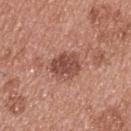Notes:
• workup · imaged on a skin check; not biopsied
• illumination · white-light
• image-analysis metrics · an area of roughly 11 mm², a shape eccentricity near 0.6, and a symmetry-axis asymmetry near 0.15; an average lesion color of about L≈49 a*≈24 b*≈27 (CIELAB), about 11 CIELAB-L* units darker than the surrounding skin, and a normalized border contrast of about 8
• location · the upper back
• acquisition · ~15 mm crop, total-body skin-cancer survey
• patient · male, aged around 55